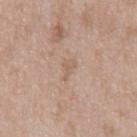Case summary:
* follow-up: catalogued during a skin exam; not biopsied
* subject: male, in their 50s
* acquisition: ~15 mm crop, total-body skin-cancer survey
* lesion diameter: ≈2.5 mm
* tile lighting: white-light
* automated metrics: a lesion area of about 2.5 mm² and an eccentricity of roughly 0.85; an average lesion color of about L≈60 a*≈17 b*≈28 (CIELAB), about 6 CIELAB-L* units darker than the surrounding skin, and a normalized border contrast of about 4.5; a nevus-likeness score of about 0/100 and a detector confidence of about 100 out of 100 that the crop contains a lesion
* site: the chest